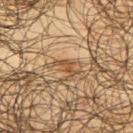Imaged during a routine full-body skin examination; the lesion was not biopsied and no histopathology is available.
A lesion tile, about 15 mm wide, cut from a 3D total-body photograph.
The subject is a male in their mid-60s.
From the right upper arm.